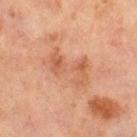Case summary:
- notes · catalogued during a skin exam; not biopsied
- acquisition · ~15 mm tile from a whole-body skin photo
- patient · male, about 65 years old
- lighting · cross-polarized